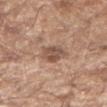No biopsy was performed on this lesion — it was imaged during a full skin examination and was not determined to be concerning. The lesion's longest dimension is about 3 mm. The lesion-visualizer software estimated a mean CIELAB color near L≈52 a*≈18 b*≈27, a lesion–skin lightness drop of about 11, and a normalized border contrast of about 7.5. It also reported border irregularity of about 2 on a 0–10 scale and a color-variation rating of about 3/10. The analysis additionally found an automated nevus-likeness rating near 5 out of 100 and a detector confidence of about 100 out of 100 that the crop contains a lesion. Cropped from a whole-body photographic skin survey; the tile spans about 15 mm. On the arm. The tile uses white-light illumination. The patient is a male aged 58 to 62.